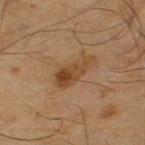  biopsy_status: not biopsied; imaged during a skin examination
  patient:
    sex: male
    age_approx: 65
  site: leg
  image:
    source: total-body photography crop
    field_of_view_mm: 15